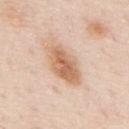Clinical impression:
This lesion was catalogued during total-body skin photography and was not selected for biopsy.
Clinical summary:
A male subject, approximately 80 years of age. This image is a 15 mm lesion crop taken from a total-body photograph. Captured under white-light illumination. The total-body-photography lesion software estimated a mean CIELAB color near L≈66 a*≈20 b*≈33, a lesion–skin lightness drop of about 12, and a lesion-to-skin contrast of about 8 (normalized; higher = more distinct). From the mid back. About 5.5 mm across.